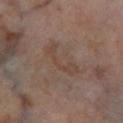  biopsy_status: not biopsied; imaged during a skin examination
  site: left lower leg
  lesion_size:
    long_diameter_mm_approx: 5.0
  patient:
    sex: male
    age_approx: 70
  image:
    source: total-body photography crop
    field_of_view_mm: 15
  lighting: cross-polarized